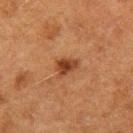This lesion was catalogued during total-body skin photography and was not selected for biopsy.
The subject is a female about 50 years old.
A 15 mm close-up extracted from a 3D total-body photography capture.
On the left thigh.